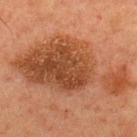Impression: Recorded during total-body skin imaging; not selected for excision or biopsy. Clinical summary: The subject is a male roughly 60 years of age. This image is a 15 mm lesion crop taken from a total-body photograph. Approximately 14 mm at its widest. The lesion is located on the upper back.